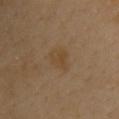  biopsy_status: not biopsied; imaged during a skin examination
  automated_metrics:
    nevus_likeness_0_100: 5
    lesion_detection_confidence_0_100: 100
  lesion_size:
    long_diameter_mm_approx: 3.0
  lighting: cross-polarized
  site: right upper arm
  patient:
    sex: male
    age_approx: 40
  image:
    source: total-body photography crop
    field_of_view_mm: 15